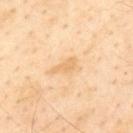A lesion tile, about 15 mm wide, cut from a 3D total-body photograph.
On the upper back.
Imaged with cross-polarized lighting.
The subject is a male roughly 55 years of age.
The recorded lesion diameter is about 3 mm.